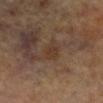Part of a total-body skin-imaging series; this lesion was reviewed on a skin check and was not flagged for biopsy. Cropped from a total-body skin-imaging series; the visible field is about 15 mm. The lesion is located on the left leg. A female patient, approximately 65 years of age. Measured at roughly 2.5 mm in maximum diameter. Captured under cross-polarized illumination.